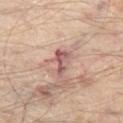<record>
<biopsy_status>not biopsied; imaged during a skin examination</biopsy_status>
<patient>
  <age_approx>55</age_approx>
</patient>
<automated_metrics>
  <area_mm2_approx>4.5</area_mm2_approx>
  <eccentricity>0.9</eccentricity>
  <shape_asymmetry>0.45</shape_asymmetry>
  <nevus_likeness_0_100>0</nevus_likeness_0_100>
  <lesion_detection_confidence_0_100>90</lesion_detection_confidence_0_100>
</automated_metrics>
<image>
  <source>total-body photography crop</source>
  <field_of_view_mm>15</field_of_view_mm>
</image>
<lesion_size>
  <long_diameter_mm_approx>3.5</long_diameter_mm_approx>
</lesion_size>
<site>leg</site>
</record>